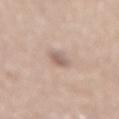<case>
  <biopsy_status>not biopsied; imaged during a skin examination</biopsy_status>
  <site>mid back</site>
  <image>
    <source>total-body photography crop</source>
    <field_of_view_mm>15</field_of_view_mm>
  </image>
  <patient>
    <sex>male</sex>
    <age_approx>75</age_approx>
  </patient>
  <lighting>white-light</lighting>
  <automated_metrics>
    <cielab_L>60</cielab_L>
    <cielab_a>16</cielab_a>
    <cielab_b>23</cielab_b>
    <vs_skin_darker_L>10.0</vs_skin_darker_L>
    <vs_skin_contrast_norm>6.5</vs_skin_contrast_norm>
    <border_irregularity_0_10>2.0</border_irregularity_0_10>
    <color_variation_0_10>2.5</color_variation_0_10>
    <peripheral_color_asymmetry>1.0</peripheral_color_asymmetry>
    <nevus_likeness_0_100>0</nevus_likeness_0_100>
    <lesion_detection_confidence_0_100>100</lesion_detection_confidence_0_100>
  </automated_metrics>
  <lesion_size>
    <long_diameter_mm_approx>3.0</long_diameter_mm_approx>
  </lesion_size>
</case>